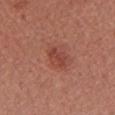The lesion was tiled from a total-body skin photograph and was not biopsied. A female subject in their mid-20s. A region of skin cropped from a whole-body photographic capture, roughly 15 mm wide. The lesion is on the chest.